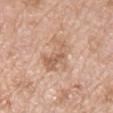patient = male, about 65 years old
diameter = about 5 mm
image = ~15 mm crop, total-body skin-cancer survey
body site = the front of the torso
tile lighting = white-light illumination